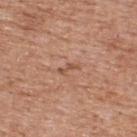Q: Was this lesion biopsied?
A: total-body-photography surveillance lesion; no biopsy
Q: Where on the body is the lesion?
A: the upper back
Q: How was this image acquired?
A: ~15 mm crop, total-body skin-cancer survey
Q: Patient demographics?
A: male, about 60 years old
Q: What is the lesion's diameter?
A: ~2.5 mm (longest diameter)